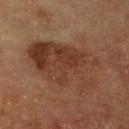follow-up = total-body-photography surveillance lesion; no biopsy | imaging modality = 15 mm crop, total-body photography | diameter = ~9 mm (longest diameter) | anatomic site = the chest | patient = male, roughly 70 years of age.